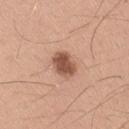{"biopsy_status": "not biopsied; imaged during a skin examination", "site": "right upper arm", "image": {"source": "total-body photography crop", "field_of_view_mm": 15}, "patient": {"sex": "male", "age_approx": 40}}